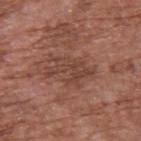Assessment:
Imaged during a routine full-body skin examination; the lesion was not biopsied and no histopathology is available.
Clinical summary:
Automated image analysis of the tile measured an outline eccentricity of about 0.85 (0 = round, 1 = elongated) and a shape-asymmetry score of about 0.5 (0 = symmetric). The analysis additionally found a lesion-to-skin contrast of about 6 (normalized; higher = more distinct). And it measured a border-irregularity index near 9/10, a within-lesion color-variation index near 2.5/10, and a peripheral color-asymmetry measure near 1. And it measured a classifier nevus-likeness of about 0/100 and a lesion-detection confidence of about 100/100. A male patient, roughly 75 years of age. A 15 mm close-up extracted from a 3D total-body photography capture. From the upper back.